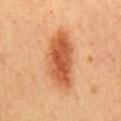Imaged with cross-polarized lighting. A 15 mm close-up extracted from a 3D total-body photography capture. On the mid back. The recorded lesion diameter is about 7.5 mm. A female patient, aged 58–62.